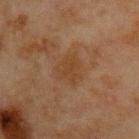<tbp_lesion>
<biopsy_status>not biopsied; imaged during a skin examination</biopsy_status>
<patient>
  <sex>male</sex>
  <age_approx>65</age_approx>
</patient>
<image>
  <source>total-body photography crop</source>
  <field_of_view_mm>15</field_of_view_mm>
</image>
<lighting>cross-polarized</lighting>
<automated_metrics>
  <cielab_L>33</cielab_L>
  <cielab_a>16</cielab_a>
  <cielab_b>28</cielab_b>
  <vs_skin_darker_L>4.0</vs_skin_darker_L>
  <vs_skin_contrast_norm>5.5</vs_skin_contrast_norm>
  <border_irregularity_0_10>3.0</border_irregularity_0_10>
  <color_variation_0_10>2.0</color_variation_0_10>
  <peripheral_color_asymmetry>0.5</peripheral_color_asymmetry>
  <nevus_likeness_0_100>0</nevus_likeness_0_100>
  <lesion_detection_confidence_0_100>100</lesion_detection_confidence_0_100>
</automated_metrics>
<site>front of the torso</site>
<lesion_size>
  <long_diameter_mm_approx>3.5</long_diameter_mm_approx>
</lesion_size>
</tbp_lesion>